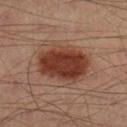The subject is a male in their 40s.
The lesion's longest dimension is about 6.5 mm.
A roughly 15 mm field-of-view crop from a total-body skin photograph.
This is a cross-polarized tile.
Located on the right lower leg.